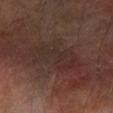{"biopsy_status": "not biopsied; imaged during a skin examination", "lesion_size": {"long_diameter_mm_approx": 6.5}, "patient": {"sex": "male", "age_approx": 70}, "site": "left forearm", "image": {"source": "total-body photography crop", "field_of_view_mm": 15}}